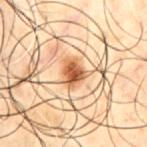No biopsy was performed on this lesion — it was imaged during a full skin examination and was not determined to be concerning. Imaged with cross-polarized lighting. A male patient approximately 50 years of age. The lesion is on the chest. This image is a 15 mm lesion crop taken from a total-body photograph. About 2.5 mm across.